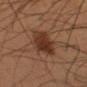Captured during whole-body skin photography for melanoma surveillance; the lesion was not biopsied.
Longest diameter approximately 5 mm.
A 15 mm close-up extracted from a 3D total-body photography capture.
Located on the left upper arm.
The tile uses cross-polarized illumination.
The subject is a male aged approximately 55.
An algorithmic analysis of the crop reported a footprint of about 13 mm², a shape eccentricity near 0.75, and two-axis asymmetry of about 0.15. The software also gave a border-irregularity rating of about 2/10.